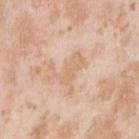  biopsy_status: not biopsied; imaged during a skin examination
  site: right upper arm
  patient:
    sex: female
    age_approx: 25
  lesion_size:
    long_diameter_mm_approx: 5.0
  image:
    source: total-body photography crop
    field_of_view_mm: 15